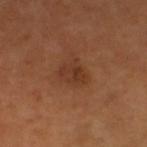No biopsy was performed on this lesion — it was imaged during a full skin examination and was not determined to be concerning. The subject is a female aged approximately 55. The lesion-visualizer software estimated a nevus-likeness score of about 10/100 and a detector confidence of about 100 out of 100 that the crop contains a lesion. Cropped from a whole-body photographic skin survey; the tile spans about 15 mm. Captured under cross-polarized illumination. The lesion is located on the left lower leg. About 2.5 mm across.